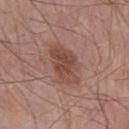follow-up: total-body-photography surveillance lesion; no biopsy | image: total-body-photography crop, ~15 mm field of view | site: the chest | size: ≈5 mm | lighting: white-light | subject: male, roughly 75 years of age.